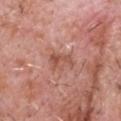This lesion was catalogued during total-body skin photography and was not selected for biopsy. Located on the head or neck. A male patient, aged approximately 60. The tile uses white-light illumination. A lesion tile, about 15 mm wide, cut from a 3D total-body photograph. The lesion's longest dimension is about 3 mm.